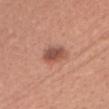Q: Was a biopsy performed?
A: total-body-photography surveillance lesion; no biopsy
Q: Automated lesion metrics?
A: a lesion–skin lightness drop of about 12 and a lesion-to-skin contrast of about 8.5 (normalized; higher = more distinct)
Q: What are the patient's age and sex?
A: female, approximately 40 years of age
Q: Lesion location?
A: the head or neck
Q: What is the imaging modality?
A: ~15 mm crop, total-body skin-cancer survey
Q: What lighting was used for the tile?
A: white-light illumination